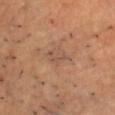workup: no biopsy performed (imaged during a skin exam)
imaging modality: 15 mm crop, total-body photography
automated metrics: a lesion area of about 2 mm², an outline eccentricity of about 0.9 (0 = round, 1 = elongated), and a shape-asymmetry score of about 0.8 (0 = symmetric); about 7 CIELAB-L* units darker than the surrounding skin and a normalized border contrast of about 5; border irregularity of about 8 on a 0–10 scale and a peripheral color-asymmetry measure near 0
body site: the head or neck
diameter: ≈2.5 mm